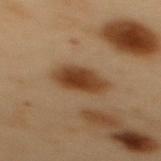workup=no biopsy performed (imaged during a skin exam); patient=male, about 55 years old; TBP lesion metrics=an average lesion color of about L≈32 a*≈16 b*≈28 (CIELAB); location=the back; image=~15 mm crop, total-body skin-cancer survey; size=≈4.5 mm; tile lighting=cross-polarized illumination.